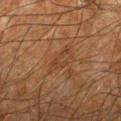{
  "biopsy_status": "not biopsied; imaged during a skin examination",
  "image": {
    "source": "total-body photography crop",
    "field_of_view_mm": 15
  },
  "lighting": "cross-polarized",
  "site": "left forearm",
  "patient": {
    "sex": "male",
    "age_approx": 65
  }
}